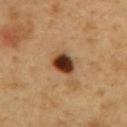Q: Is there a histopathology result?
A: imaged on a skin check; not biopsied
Q: Patient demographics?
A: male, in their 50s
Q: What lighting was used for the tile?
A: cross-polarized illumination
Q: How was this image acquired?
A: 15 mm crop, total-body photography
Q: What is the lesion's diameter?
A: ~3 mm (longest diameter)
Q: Lesion location?
A: the mid back
Q: What did automated image analysis measure?
A: a border-irregularity rating of about 1.5/10, internal color variation of about 6 on a 0–10 scale, and peripheral color asymmetry of about 1.5